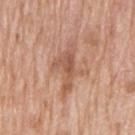Impression:
Part of a total-body skin-imaging series; this lesion was reviewed on a skin check and was not flagged for biopsy.
Context:
A male patient, roughly 70 years of age. A 15 mm crop from a total-body photograph taken for skin-cancer surveillance. An algorithmic analysis of the crop reported a mean CIELAB color near L≈56 a*≈22 b*≈31, a lesion–skin lightness drop of about 9, and a lesion-to-skin contrast of about 6.5 (normalized; higher = more distinct). The lesion is on the arm. Imaged with white-light lighting. Measured at roughly 6 mm in maximum diameter.